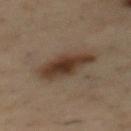No biopsy was performed on this lesion — it was imaged during a full skin examination and was not determined to be concerning.
A region of skin cropped from a whole-body photographic capture, roughly 15 mm wide.
On the mid back.
A male patient aged around 55.